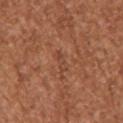This lesion was catalogued during total-body skin photography and was not selected for biopsy. From the right upper arm. The recorded lesion diameter is about 3 mm. Cropped from a whole-body photographic skin survey; the tile spans about 15 mm. The patient is a female aged 38 to 42. An algorithmic analysis of the crop reported a lesion area of about 2.5 mm² and an eccentricity of roughly 0.95. The analysis additionally found a lesion-to-skin contrast of about 4.5 (normalized; higher = more distinct).